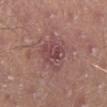The lesion was photographed on a routine skin check and not biopsied; there is no pathology result. The subject is a male roughly 40 years of age. A roughly 15 mm field-of-view crop from a total-body skin photograph. From the right lower leg.